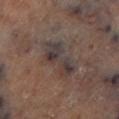<lesion>
  <biopsy_status>not biopsied; imaged during a skin examination</biopsy_status>
  <lesion_size>
    <long_diameter_mm_approx>5.5</long_diameter_mm_approx>
  </lesion_size>
  <site>left lower leg</site>
  <image>
    <source>total-body photography crop</source>
    <field_of_view_mm>15</field_of_view_mm>
  </image>
  <lighting>cross-polarized</lighting>
  <automated_metrics>
    <vs_skin_darker_L>8.0</vs_skin_darker_L>
    <vs_skin_contrast_norm>8.5</vs_skin_contrast_norm>
    <border_irregularity_0_10>5.5</border_irregularity_0_10>
    <color_variation_0_10>7.5</color_variation_0_10>
  </automated_metrics>
</lesion>